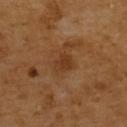Assessment: This lesion was catalogued during total-body skin photography and was not selected for biopsy. Clinical summary: A female patient, in their mid-50s. On the upper back. This is a cross-polarized tile. Longest diameter approximately 2.5 mm. A 15 mm crop from a total-body photograph taken for skin-cancer surveillance. Automated image analysis of the tile measured a footprint of about 4 mm², an eccentricity of roughly 0.6, and a shape-asymmetry score of about 0.25 (0 = symmetric). The analysis additionally found a border-irregularity rating of about 2/10 and radial color variation of about 0.5. The analysis additionally found a classifier nevus-likeness of about 0/100 and lesion-presence confidence of about 100/100.